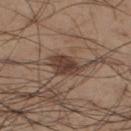The lesion was tiled from a total-body skin photograph and was not biopsied. The lesion is located on the left lower leg. The lesion-visualizer software estimated a lesion color around L≈40 a*≈17 b*≈24 in CIELAB and a normalized border contrast of about 9.5. The analysis additionally found a nevus-likeness score of about 90/100 and lesion-presence confidence of about 100/100. A 15 mm close-up tile from a total-body photography series done for melanoma screening. A male patient, in their mid-50s. The lesion's longest dimension is about 4 mm.